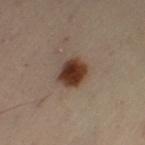follow-up: total-body-photography surveillance lesion; no biopsy | location: the left thigh | tile lighting: cross-polarized | lesion size: ~3.5 mm (longest diameter) | subject: female, about 50 years old | image source: total-body-photography crop, ~15 mm field of view.